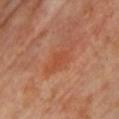Part of a total-body skin-imaging series; this lesion was reviewed on a skin check and was not flagged for biopsy. A female subject aged around 65. A lesion tile, about 15 mm wide, cut from a 3D total-body photograph. This is a cross-polarized tile. The lesion-visualizer software estimated a lesion area of about 3.5 mm², a shape eccentricity near 0.8, and a shape-asymmetry score of about 0.4 (0 = symmetric). The software also gave a border-irregularity rating of about 3.5/10. It also reported a classifier nevus-likeness of about 10/100 and a detector confidence of about 100 out of 100 that the crop contains a lesion. Located on the chest. Longest diameter approximately 3 mm.